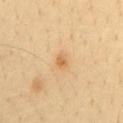Q: Is there a histopathology result?
A: catalogued during a skin exam; not biopsied
Q: How was this image acquired?
A: total-body-photography crop, ~15 mm field of view
Q: Lesion location?
A: the mid back
Q: Who is the patient?
A: male, roughly 45 years of age